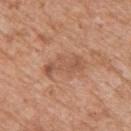Notes:
* notes — catalogued during a skin exam; not biopsied
* size — about 5 mm
* TBP lesion metrics — an automated nevus-likeness rating near 5 out of 100 and a detector confidence of about 100 out of 100 that the crop contains a lesion
* body site — the upper back
* acquisition — total-body-photography crop, ~15 mm field of view
* patient — male, aged 58 to 62